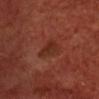Clinical impression:
This lesion was catalogued during total-body skin photography and was not selected for biopsy.
Background:
A male patient aged 68–72. Cropped from a total-body skin-imaging series; the visible field is about 15 mm. About 3 mm across. On the chest.